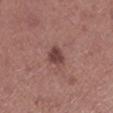| key | value |
|---|---|
| biopsy status | catalogued during a skin exam; not biopsied |
| location | the left lower leg |
| imaging modality | ~15 mm crop, total-body skin-cancer survey |
| patient | female, aged 63 to 67 |